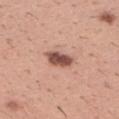| feature | finding |
|---|---|
| notes | catalogued during a skin exam; not biopsied |
| patient | male, approximately 40 years of age |
| image source | 15 mm crop, total-body photography |
| location | the arm |